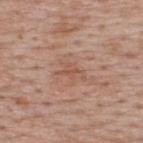The lesion was tiled from a total-body skin photograph and was not biopsied. Cropped from a whole-body photographic skin survey; the tile spans about 15 mm. Captured under white-light illumination. From the upper back. A male patient about 65 years old.